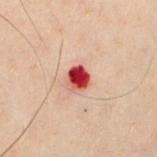biopsy status: no biopsy performed (imaged during a skin exam); image: ~15 mm tile from a whole-body skin photo; lighting: cross-polarized illumination; anatomic site: the chest; size: ≈2.5 mm; subject: male, in their 50s.